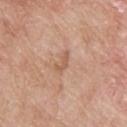Assessment: The lesion was tiled from a total-body skin photograph and was not biopsied. Context: This image is a 15 mm lesion crop taken from a total-body photograph. Imaged with white-light lighting. The patient is a male about 65 years old. The lesion is located on the back.